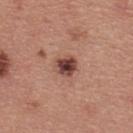The lesion was tiled from a total-body skin photograph and was not biopsied. Measured at roughly 3 mm in maximum diameter. The tile uses white-light illumination. The lesion is on the upper back. A male subject, aged 38–42. A close-up tile cropped from a whole-body skin photograph, about 15 mm across. An algorithmic analysis of the crop reported a color-variation rating of about 5/10. The analysis additionally found an automated nevus-likeness rating near 75 out of 100 and lesion-presence confidence of about 100/100.